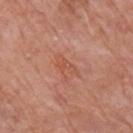Imaged during a routine full-body skin examination; the lesion was not biopsied and no histopathology is available. The recorded lesion diameter is about 3 mm. A male subject, aged approximately 80. The lesion is located on the right upper arm. Imaged with white-light lighting. A lesion tile, about 15 mm wide, cut from a 3D total-body photograph.